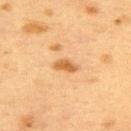Clinical impression:
No biopsy was performed on this lesion — it was imaged during a full skin examination and was not determined to be concerning.
Background:
The lesion-visualizer software estimated a footprint of about 3 mm² and a symmetry-axis asymmetry near 0.35. The software also gave a normalized lesion–skin contrast near 8. The analysis additionally found a border-irregularity index near 3/10, a color-variation rating of about 1.5/10, and a peripheral color-asymmetry measure near 0.5. The tile uses cross-polarized illumination. The lesion is located on the upper back. The lesion's longest dimension is about 2.5 mm. The patient is a female approximately 40 years of age. A region of skin cropped from a whole-body photographic capture, roughly 15 mm wide.